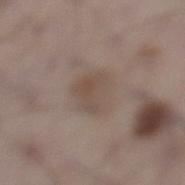notes = total-body-photography surveillance lesion; no biopsy | illumination = white-light illumination | body site = the left lower leg | subject = male, roughly 70 years of age | acquisition = ~15 mm crop, total-body skin-cancer survey.